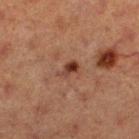Assessment: Captured during whole-body skin photography for melanoma surveillance; the lesion was not biopsied. Image and clinical context: The lesion is on the left thigh. Longest diameter approximately 3 mm. A 15 mm crop from a total-body photograph taken for skin-cancer surveillance. Automated image analysis of the tile measured a border-irregularity index near 3/10. The tile uses cross-polarized illumination. A female patient approximately 40 years of age.